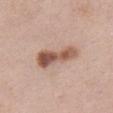Assessment:
Recorded during total-body skin imaging; not selected for excision or biopsy.
Context:
A 15 mm close-up tile from a total-body photography series done for melanoma screening. From the left thigh. A female patient in their 40s.